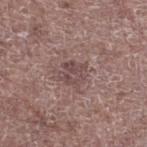follow-up=imaged on a skin check; not biopsied
lighting=white-light illumination
automated lesion analysis=a footprint of about 6 mm², an eccentricity of roughly 0.4, and two-axis asymmetry of about 0.25
imaging modality=total-body-photography crop, ~15 mm field of view
body site=the left lower leg
subject=male, aged around 70
lesion size=≈3 mm